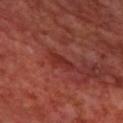tile lighting: cross-polarized illumination
lesion diameter: ~3 mm (longest diameter)
anatomic site: the upper back
imaging modality: total-body-photography crop, ~15 mm field of view
patient: male, aged 68–72